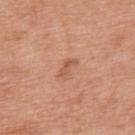Assessment: Part of a total-body skin-imaging series; this lesion was reviewed on a skin check and was not flagged for biopsy. Clinical summary: A male patient in their 70s. The lesion is located on the mid back. A 15 mm close-up tile from a total-body photography series done for melanoma screening.